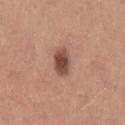Imaged during a routine full-body skin examination; the lesion was not biopsied and no histopathology is available.
On the right thigh.
A close-up tile cropped from a whole-body skin photograph, about 15 mm across.
The patient is a female in their mid- to late 20s.